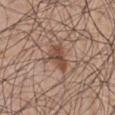<record>
<biopsy_status>not biopsied; imaged during a skin examination</biopsy_status>
<patient>
  <sex>male</sex>
  <age_approx>55</age_approx>
</patient>
<image>
  <source>total-body photography crop</source>
  <field_of_view_mm>15</field_of_view_mm>
</image>
<site>chest</site>
</record>